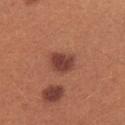notes: total-body-photography surveillance lesion; no biopsy | anatomic site: the right forearm | diameter: about 3.5 mm | TBP lesion metrics: border irregularity of about 2 on a 0–10 scale and peripheral color asymmetry of about 0.5; a nevus-likeness score of about 90/100 and lesion-presence confidence of about 100/100 | subject: female, approximately 25 years of age | imaging modality: 15 mm crop, total-body photography.